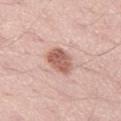Assessment: The lesion was photographed on a routine skin check and not biopsied; there is no pathology result. Image and clinical context: A roughly 15 mm field-of-view crop from a total-body skin photograph. A male subject, about 50 years old. The lesion-visualizer software estimated an area of roughly 7.5 mm² and an outline eccentricity of about 0.7 (0 = round, 1 = elongated). The software also gave a classifier nevus-likeness of about 70/100 and a detector confidence of about 100 out of 100 that the crop contains a lesion. Approximately 3.5 mm at its widest. Located on the left thigh. This is a white-light tile.